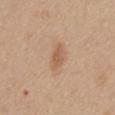Impression:
Recorded during total-body skin imaging; not selected for excision or biopsy.
Context:
An algorithmic analysis of the crop reported a lesion area of about 3.5 mm², a shape eccentricity near 0.9, and a shape-asymmetry score of about 0.2 (0 = symmetric). The analysis additionally found a lesion color around L≈57 a*≈19 b*≈32 in CIELAB, roughly 9 lightness units darker than nearby skin, and a normalized lesion–skin contrast near 6.5. The analysis additionally found lesion-presence confidence of about 100/100. A male patient roughly 30 years of age. Imaged with white-light lighting. A 15 mm close-up tile from a total-body photography series done for melanoma screening. Located on the abdomen.